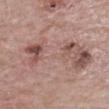This image is a 15 mm lesion crop taken from a total-body photograph.
The subject is a male aged around 85 or older.
On the chest.